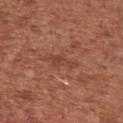| field | value |
|---|---|
| workup | total-body-photography surveillance lesion; no biopsy |
| lesion size | ~4 mm (longest diameter) |
| anatomic site | the front of the torso |
| image | total-body-photography crop, ~15 mm field of view |
| patient | male, about 45 years old |
| tile lighting | white-light |
| image-analysis metrics | a lesion area of about 4.5 mm² and an outline eccentricity of about 0.95 (0 = round, 1 = elongated); border irregularity of about 6 on a 0–10 scale, a within-lesion color-variation index near 1/10, and radial color variation of about 0.5 |